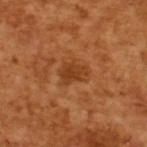Q: Is there a histopathology result?
A: no biopsy performed (imaged during a skin exam)
Q: What are the patient's age and sex?
A: male, aged 63–67
Q: Automated lesion metrics?
A: an area of roughly 5.5 mm², an outline eccentricity of about 0.7 (0 = round, 1 = elongated), and a symmetry-axis asymmetry near 0.3; a nevus-likeness score of about 20/100
Q: What is the imaging modality?
A: ~15 mm crop, total-body skin-cancer survey
Q: Lesion size?
A: about 3 mm
Q: What lighting was used for the tile?
A: cross-polarized illumination